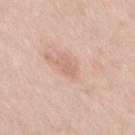biopsy_status: not biopsied; imaged during a skin examination
lighting: white-light
site: mid back
lesion_size:
  long_diameter_mm_approx: 1.5
patient:
  sex: male
  age_approx: 35
image:
  source: total-body photography crop
  field_of_view_mm: 15